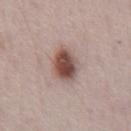Recorded during total-body skin imaging; not selected for excision or biopsy. A close-up tile cropped from a whole-body skin photograph, about 15 mm across. From the abdomen. The subject is a male roughly 70 years of age.